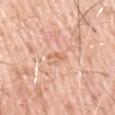Q: Was a biopsy performed?
A: catalogued during a skin exam; not biopsied
Q: Where on the body is the lesion?
A: the left upper arm
Q: What is the lesion's diameter?
A: about 2.5 mm
Q: Automated lesion metrics?
A: a shape eccentricity near 0.85; a mean CIELAB color near L≈66 a*≈23 b*≈33 and a normalized border contrast of about 5; a border-irregularity index near 5.5/10, a color-variation rating of about 0/10, and radial color variation of about 0; an automated nevus-likeness rating near 0 out of 100
Q: Patient demographics?
A: male, aged approximately 70
Q: What lighting was used for the tile?
A: white-light illumination
Q: What is the imaging modality?
A: 15 mm crop, total-body photography